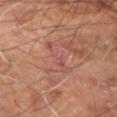{
  "biopsy_status": "not biopsied; imaged during a skin examination",
  "site": "right arm",
  "lesion_size": {
    "long_diameter_mm_approx": 4.5
  },
  "lighting": "cross-polarized",
  "automated_metrics": {
    "cielab_L": 49,
    "cielab_a": 23,
    "cielab_b": 25,
    "vs_skin_darker_L": 6.0,
    "vs_skin_contrast_norm": 5.0
  },
  "patient": {
    "sex": "male",
    "age_approx": 60
  },
  "image": {
    "source": "total-body photography crop",
    "field_of_view_mm": 15
  }
}